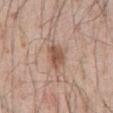Impression: The lesion was photographed on a routine skin check and not biopsied; there is no pathology result. Image and clinical context: Imaged with white-light lighting. A male patient, aged 53–57. Automated image analysis of the tile measured a symmetry-axis asymmetry near 0.25. The analysis additionally found a border-irregularity index near 2.5/10, a color-variation rating of about 2.5/10, and a peripheral color-asymmetry measure near 1. From the abdomen. The recorded lesion diameter is about 3 mm. A roughly 15 mm field-of-view crop from a total-body skin photograph.